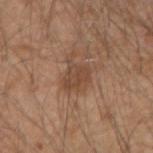<case>
  <biopsy_status>not biopsied; imaged during a skin examination</biopsy_status>
  <patient>
    <sex>male</sex>
    <age_approx>50</age_approx>
  </patient>
  <lesion_size>
    <long_diameter_mm_approx>4.0</long_diameter_mm_approx>
  </lesion_size>
  <lighting>white-light</lighting>
  <automated_metrics>
    <eccentricity>0.6</eccentricity>
    <shape_asymmetry>0.35</shape_asymmetry>
    <cielab_L>46</cielab_L>
    <cielab_a>19</cielab_a>
    <cielab_b>30</cielab_b>
    <vs_skin_darker_L>8.0</vs_skin_darker_L>
    <vs_skin_contrast_norm>6.5</vs_skin_contrast_norm>
    <border_irregularity_0_10>4.5</border_irregularity_0_10>
    <color_variation_0_10>2.5</color_variation_0_10>
    <peripheral_color_asymmetry>1.0</peripheral_color_asymmetry>
    <nevus_likeness_0_100>35</nevus_likeness_0_100>
  </automated_metrics>
  <image>
    <source>total-body photography crop</source>
    <field_of_view_mm>15</field_of_view_mm>
  </image>
  <site>right upper arm</site>
</case>